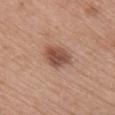Findings:
• biopsy status · total-body-photography surveillance lesion; no biopsy
• lesion diameter · ≈4 mm
• site · the right upper arm
• illumination · white-light
• image source · 15 mm crop, total-body photography
• patient · female, aged 63–67